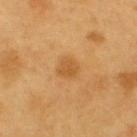Case summary:
– biopsy status — total-body-photography surveillance lesion; no biopsy
– patient — male, aged 58–62
– imaging modality — ~15 mm crop, total-body skin-cancer survey
– site — the upper back
– illumination — cross-polarized
– diameter — ~2.5 mm (longest diameter)
– automated metrics — an area of roughly 5 mm² and two-axis asymmetry of about 0.2; an average lesion color of about L≈55 a*≈22 b*≈45 (CIELAB) and a lesion–skin lightness drop of about 8; border irregularity of about 1.5 on a 0–10 scale; an automated nevus-likeness rating near 60 out of 100 and a detector confidence of about 100 out of 100 that the crop contains a lesion